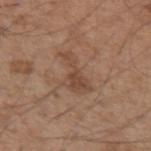Background: This is a white-light tile. On the arm. A region of skin cropped from a whole-body photographic capture, roughly 15 mm wide. A male subject aged approximately 55. About 5 mm across.